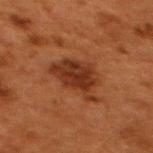The lesion was tiled from a total-body skin photograph and was not biopsied. A female patient in their 50s. The tile uses cross-polarized illumination. Cropped from a total-body skin-imaging series; the visible field is about 15 mm. Located on the upper back. Approximately 5.5 mm at its widest. An algorithmic analysis of the crop reported an automated nevus-likeness rating near 55 out of 100.